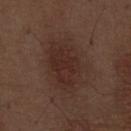Notes:
* biopsy status · total-body-photography surveillance lesion; no biopsy
* site · the abdomen
* illumination · white-light illumination
* patient · male, aged approximately 70
* diameter · about 7 mm
* automated metrics · an area of roughly 23 mm², a shape eccentricity near 0.8, and two-axis asymmetry of about 0.15; a lesion–skin lightness drop of about 6 and a normalized lesion–skin contrast near 6.5; a within-lesion color-variation index near 2.5/10; a nevus-likeness score of about 55/100 and a detector confidence of about 100 out of 100 that the crop contains a lesion
* image source · ~15 mm tile from a whole-body skin photo